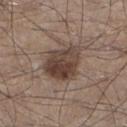notes = total-body-photography surveillance lesion; no biopsy | diameter = ≈5.5 mm | location = the leg | acquisition = ~15 mm crop, total-body skin-cancer survey | subject = male, about 45 years old.